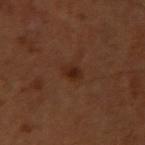Notes:
- biopsy status: imaged on a skin check; not biopsied
- lighting: cross-polarized illumination
- image source: total-body-photography crop, ~15 mm field of view
- anatomic site: the right upper arm
- subject: male, aged around 55
- diameter: ≈2.5 mm
- TBP lesion metrics: a footprint of about 3.5 mm² and an outline eccentricity of about 0.8 (0 = round, 1 = elongated); an average lesion color of about L≈25 a*≈20 b*≈27 (CIELAB), a lesion–skin lightness drop of about 7, and a normalized lesion–skin contrast near 7.5; border irregularity of about 2 on a 0–10 scale, a color-variation rating of about 1.5/10, and peripheral color asymmetry of about 0.5; an automated nevus-likeness rating near 85 out of 100 and a detector confidence of about 100 out of 100 that the crop contains a lesion